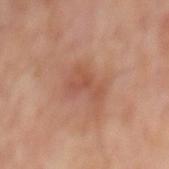{
  "biopsy_status": "not biopsied; imaged during a skin examination",
  "lesion_size": {
    "long_diameter_mm_approx": 4.0
  },
  "lighting": "cross-polarized",
  "automated_metrics": {
    "cielab_L": 49,
    "cielab_a": 23,
    "cielab_b": 29,
    "vs_skin_darker_L": 7.0,
    "vs_skin_contrast_norm": 5.0,
    "border_irregularity_0_10": 6.5,
    "color_variation_0_10": 2.5,
    "peripheral_color_asymmetry": 1.0
  },
  "image": {
    "source": "total-body photography crop",
    "field_of_view_mm": 15
  },
  "patient": {
    "sex": "male",
    "age_approx": 65
  },
  "site": "mid back"
}